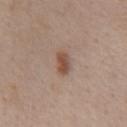Q: Is there a histopathology result?
A: catalogued during a skin exam; not biopsied
Q: What lighting was used for the tile?
A: white-light
Q: What is the lesion's diameter?
A: ~2.5 mm (longest diameter)
Q: Who is the patient?
A: male, aged 58 to 62
Q: What kind of image is this?
A: 15 mm crop, total-body photography
Q: Where on the body is the lesion?
A: the front of the torso